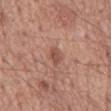The lesion was tiled from a total-body skin photograph and was not biopsied. Captured under white-light illumination. Cropped from a total-body skin-imaging series; the visible field is about 15 mm. The patient is a male approximately 55 years of age. From the mid back. The recorded lesion diameter is about 2.5 mm.